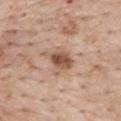Part of a total-body skin-imaging series; this lesion was reviewed on a skin check and was not flagged for biopsy. Cropped from a whole-body photographic skin survey; the tile spans about 15 mm. The lesion is located on the mid back. A male patient, roughly 60 years of age. The lesion-visualizer software estimated a lesion color around L≈54 a*≈20 b*≈29 in CIELAB, roughly 13 lightness units darker than nearby skin, and a normalized border contrast of about 9. And it measured a border-irregularity rating of about 3/10 and a color-variation rating of about 5.5/10. The lesion's longest dimension is about 3 mm. The tile uses white-light illumination.